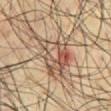biopsy status — no biopsy performed (imaged during a skin exam); subject — male, approximately 60 years of age; location — the front of the torso; acquisition — total-body-photography crop, ~15 mm field of view.